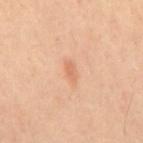The lesion was tiled from a total-body skin photograph and was not biopsied. A male patient approximately 40 years of age. From the mid back. A 15 mm close-up extracted from a 3D total-body photography capture.